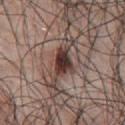<record>
  <automated_metrics>
    <area_mm2_approx>5.5</area_mm2_approx>
    <eccentricity>0.8</eccentricity>
    <border_irregularity_0_10>2.5</border_irregularity_0_10>
    <color_variation_0_10>4.5</color_variation_0_10>
    <peripheral_color_asymmetry>1.0</peripheral_color_asymmetry>
  </automated_metrics>
  <site>chest</site>
  <lighting>white-light</lighting>
  <image>
    <source>total-body photography crop</source>
    <field_of_view_mm>15</field_of_view_mm>
  </image>
  <lesion_size>
    <long_diameter_mm_approx>3.5</long_diameter_mm_approx>
  </lesion_size>
  <patient>
    <sex>male</sex>
    <age_approx>70</age_approx>
  </patient>
</record>